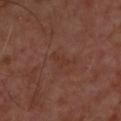Assessment:
No biopsy was performed on this lesion — it was imaged during a full skin examination and was not determined to be concerning.
Image and clinical context:
A male patient aged 68–72. A 15 mm close-up tile from a total-body photography series done for melanoma screening. The recorded lesion diameter is about 3 mm. The lesion is located on the upper back.